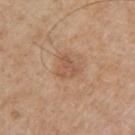Imaged during a routine full-body skin examination; the lesion was not biopsied and no histopathology is available.
Imaged with white-light lighting.
Measured at roughly 2.5 mm in maximum diameter.
A male subject, approximately 60 years of age.
A 15 mm close-up tile from a total-body photography series done for melanoma screening.
Automated tile analysis of the lesion measured a border-irregularity index near 6/10 and peripheral color asymmetry of about 0.
On the left upper arm.